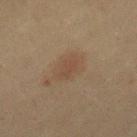Impression:
This lesion was catalogued during total-body skin photography and was not selected for biopsy.
Clinical summary:
Captured under cross-polarized illumination. A roughly 15 mm field-of-view crop from a total-body skin photograph. The patient is a female in their 40s. The lesion is on the right thigh.